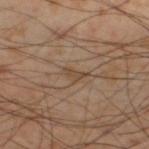biopsy_status: not biopsied; imaged during a skin examination
automated_metrics:
  area_mm2_approx: 3.0
  eccentricity: 0.9
  shape_asymmetry: 0.5
  cielab_L: 45
  cielab_a: 15
  cielab_b: 29
  vs_skin_darker_L: 7.0
  vs_skin_contrast_norm: 6.0
  border_irregularity_0_10: 5.0
  color_variation_0_10: 0.0
lighting: cross-polarized
image:
  source: total-body photography crop
  field_of_view_mm: 15
site: left lower leg
patient:
  sex: male
  age_approx: 55
lesion_size:
  long_diameter_mm_approx: 3.0